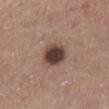* follow-up · no biopsy performed (imaged during a skin exam)
* anatomic site · the right lower leg
* patient · female, in their mid- to late 60s
* lesion diameter · ~3.5 mm (longest diameter)
* image · ~15 mm tile from a whole-body skin photo
* image-analysis metrics · a footprint of about 8.5 mm², an outline eccentricity of about 0.55 (0 = round, 1 = elongated), and a symmetry-axis asymmetry near 0.1; a mean CIELAB color near L≈40 a*≈18 b*≈21 and roughly 17 lightness units darker than nearby skin